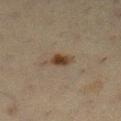– workup: total-body-photography surveillance lesion; no biopsy
– imaging modality: ~15 mm tile from a whole-body skin photo
– TBP lesion metrics: border irregularity of about 2.5 on a 0–10 scale, a color-variation rating of about 3/10, and radial color variation of about 1; a classifier nevus-likeness of about 100/100 and a detector confidence of about 100 out of 100 that the crop contains a lesion
– tile lighting: cross-polarized illumination
– patient: female, roughly 40 years of age
– location: the left lower leg
– size: ≈3 mm